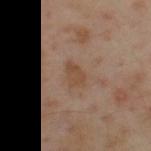Q: Was this lesion biopsied?
A: catalogued during a skin exam; not biopsied
Q: How large is the lesion?
A: about 2.5 mm
Q: What kind of image is this?
A: total-body-photography crop, ~15 mm field of view
Q: Patient demographics?
A: male, aged 53–57
Q: What lighting was used for the tile?
A: cross-polarized illumination
Q: What did automated image analysis measure?
A: an area of roughly 4 mm²; an average lesion color of about L≈46 a*≈18 b*≈30 (CIELAB), a lesion–skin lightness drop of about 7, and a normalized border contrast of about 6.5
Q: Where on the body is the lesion?
A: the upper back